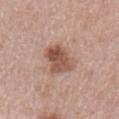Impression: Part of a total-body skin-imaging series; this lesion was reviewed on a skin check and was not flagged for biopsy. Background: A female subject, about 50 years old. A lesion tile, about 15 mm wide, cut from a 3D total-body photograph. The lesion is on the left upper arm.